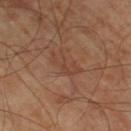{"biopsy_status": "not biopsied; imaged during a skin examination", "lighting": "cross-polarized", "lesion_size": {"long_diameter_mm_approx": 4.0}, "site": "leg", "image": {"source": "total-body photography crop", "field_of_view_mm": 15}, "patient": {"age_approx": 65}}